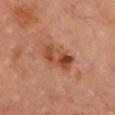diameter: ~4.5 mm (longest diameter) | body site: the front of the torso | image-analysis metrics: a mean CIELAB color near L≈48 a*≈26 b*≈34 and a lesion-to-skin contrast of about 8 (normalized; higher = more distinct); an automated nevus-likeness rating near 65 out of 100 and lesion-presence confidence of about 100/100 | image: total-body-photography crop, ~15 mm field of view | patient: male, roughly 65 years of age | illumination: cross-polarized.